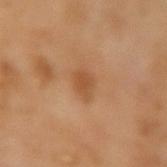Notes:
* image-analysis metrics · a lesion color around L≈50 a*≈22 b*≈37 in CIELAB and a lesion-to-skin contrast of about 6.5 (normalized; higher = more distinct); border irregularity of about 2.5 on a 0–10 scale; a classifier nevus-likeness of about 15/100 and lesion-presence confidence of about 100/100
* illumination · cross-polarized
* subject · male, about 65 years old
* diameter · ~3 mm (longest diameter)
* acquisition · 15 mm crop, total-body photography